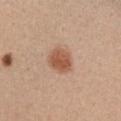notes: catalogued during a skin exam; not biopsied
TBP lesion metrics: a lesion area of about 7.5 mm² and a shape-asymmetry score of about 0.15 (0 = symmetric); about 12 CIELAB-L* units darker than the surrounding skin and a lesion-to-skin contrast of about 8.5 (normalized; higher = more distinct); a classifier nevus-likeness of about 100/100 and a detector confidence of about 100 out of 100 that the crop contains a lesion
anatomic site: the chest
diameter: ~3 mm (longest diameter)
image: total-body-photography crop, ~15 mm field of view
patient: female, in their mid- to late 30s